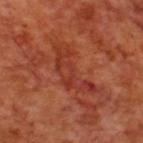This lesion was catalogued during total-body skin photography and was not selected for biopsy. A 15 mm crop from a total-body photograph taken for skin-cancer surveillance. The recorded lesion diameter is about 7.5 mm. A male patient, aged approximately 70. The lesion is on the back.